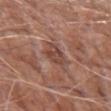Imaged during a routine full-body skin examination; the lesion was not biopsied and no histopathology is available.
Longest diameter approximately 3.5 mm.
Located on the arm.
Automated image analysis of the tile measured a lesion color around L≈45 a*≈22 b*≈26 in CIELAB, a lesion–skin lightness drop of about 9, and a lesion-to-skin contrast of about 7 (normalized; higher = more distinct). It also reported lesion-presence confidence of about 90/100.
A 15 mm close-up tile from a total-body photography series done for melanoma screening.
The patient is a male roughly 65 years of age.
The tile uses white-light illumination.